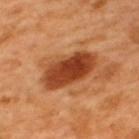Captured during whole-body skin photography for melanoma surveillance; the lesion was not biopsied. About 7 mm across. A region of skin cropped from a whole-body photographic capture, roughly 15 mm wide. A male patient aged 48 to 52. The lesion-visualizer software estimated a lesion color around L≈44 a*≈30 b*≈39 in CIELAB and a lesion-to-skin contrast of about 11.5 (normalized; higher = more distinct). The analysis additionally found a color-variation rating of about 5.5/10 and radial color variation of about 1.5. The analysis additionally found a classifier nevus-likeness of about 100/100. From the upper back.